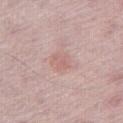Impression: Captured during whole-body skin photography for melanoma surveillance; the lesion was not biopsied. Acquisition and patient details: A male subject in their mid- to late 70s. Captured under white-light illumination. A roughly 15 mm field-of-view crop from a total-body skin photograph. The lesion is located on the right thigh. Measured at roughly 2.5 mm in maximum diameter.